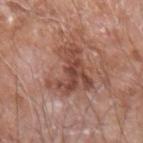Impression:
Recorded during total-body skin imaging; not selected for excision or biopsy.
Image and clinical context:
A male subject approximately 60 years of age. This is a white-light tile. A region of skin cropped from a whole-body photographic capture, roughly 15 mm wide. The total-body-photography lesion software estimated an area of roughly 16 mm², an outline eccentricity of about 0.75 (0 = round, 1 = elongated), and a symmetry-axis asymmetry near 0.55. It also reported a border-irregularity index near 7/10, a color-variation rating of about 5/10, and peripheral color asymmetry of about 1.5. The software also gave a nevus-likeness score of about 5/100 and a detector confidence of about 100 out of 100 that the crop contains a lesion. On the left forearm.